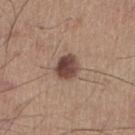Impression: Part of a total-body skin-imaging series; this lesion was reviewed on a skin check and was not flagged for biopsy. Context: A male subject, aged around 55. Located on the right lower leg. About 3 mm across. A 15 mm close-up extracted from a 3D total-body photography capture. Captured under white-light illumination. The total-body-photography lesion software estimated a footprint of about 6.5 mm². The analysis additionally found a lesion color around L≈44 a*≈19 b*≈23 in CIELAB, about 15 CIELAB-L* units darker than the surrounding skin, and a normalized border contrast of about 11.5. The analysis additionally found a nevus-likeness score of about 95/100 and a lesion-detection confidence of about 100/100.